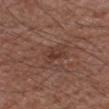The lesion was photographed on a routine skin check and not biopsied; there is no pathology result.